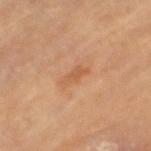follow-up: total-body-photography surveillance lesion; no biopsy | image source: ~15 mm crop, total-body skin-cancer survey | automated metrics: an average lesion color of about L≈55 a*≈22 b*≈36 (CIELAB), a lesion–skin lightness drop of about 7, and a lesion-to-skin contrast of about 5.5 (normalized; higher = more distinct); border irregularity of about 3 on a 0–10 scale, a within-lesion color-variation index near 0.5/10, and a peripheral color-asymmetry measure near 0; a nevus-likeness score of about 0/100 and a lesion-detection confidence of about 100/100 | lighting: cross-polarized illumination | patient: female, roughly 70 years of age | anatomic site: the leg.